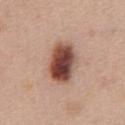No biopsy was performed on this lesion — it was imaged during a full skin examination and was not determined to be concerning.
On the chest.
A roughly 15 mm field-of-view crop from a total-body skin photograph.
The subject is a male aged 48–52.